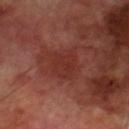biopsy status: no biopsy performed (imaged during a skin exam) | location: the right lower leg | image: total-body-photography crop, ~15 mm field of view | lighting: cross-polarized illumination | subject: male, aged around 70.